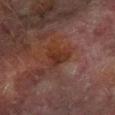<tbp_lesion>
  <biopsy_status>not biopsied; imaged during a skin examination</biopsy_status>
  <image>
    <source>total-body photography crop</source>
    <field_of_view_mm>15</field_of_view_mm>
  </image>
  <patient>
    <sex>male</sex>
    <age_approx>75</age_approx>
  </patient>
  <site>left forearm</site>
  <lighting>cross-polarized</lighting>
  <automated_metrics>
    <nevus_likeness_0_100>0</nevus_likeness_0_100>
  </automated_metrics>
  <lesion_size>
    <long_diameter_mm_approx>3.0</long_diameter_mm_approx>
  </lesion_size>
</tbp_lesion>